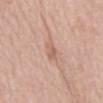diameter = about 3 mm | anatomic site = the mid back | image = ~15 mm crop, total-body skin-cancer survey | subject = female, aged 68 to 72 | illumination = white-light.